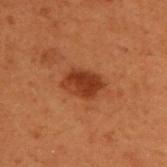| key | value |
|---|---|
| biopsy status | catalogued during a skin exam; not biopsied |
| body site | the upper back |
| lighting | cross-polarized |
| patient | male, aged 48 to 52 |
| image | ~15 mm crop, total-body skin-cancer survey |
| automated metrics | a mean CIELAB color near L≈30 a*≈24 b*≈30, about 10 CIELAB-L* units darker than the surrounding skin, and a normalized lesion–skin contrast near 9; a nevus-likeness score of about 95/100 and a detector confidence of about 100 out of 100 that the crop contains a lesion |
| lesion diameter | ~4 mm (longest diameter) |